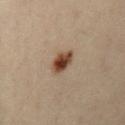A lesion tile, about 15 mm wide, cut from a 3D total-body photograph. The lesion is on the abdomen. A female patient in their 30s.